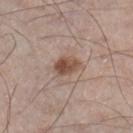Recorded during total-body skin imaging; not selected for excision or biopsy. Automated tile analysis of the lesion measured an area of roughly 6 mm², an eccentricity of roughly 0.8, and a shape-asymmetry score of about 0.25 (0 = symmetric). And it measured a mean CIELAB color near L≈50 a*≈19 b*≈27, a lesion–skin lightness drop of about 12, and a normalized border contrast of about 9. The software also gave an automated nevus-likeness rating near 90 out of 100 and a lesion-detection confidence of about 100/100. A male subject about 60 years old. From the right lower leg. Longest diameter approximately 3.5 mm. A roughly 15 mm field-of-view crop from a total-body skin photograph.